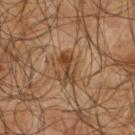The subject is a male aged around 65. The lesion is located on the upper back. A 15 mm crop from a total-body photograph taken for skin-cancer surveillance. Longest diameter approximately 4 mm.